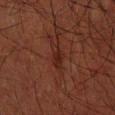Captured during whole-body skin photography for melanoma surveillance; the lesion was not biopsied.
The lesion is located on the right forearm.
A male patient in their 60s.
Captured under cross-polarized illumination.
A 15 mm crop from a total-body photograph taken for skin-cancer surveillance.
Automated image analysis of the tile measured an area of roughly 4.5 mm², an outline eccentricity of about 0.85 (0 = round, 1 = elongated), and two-axis asymmetry of about 0.35. It also reported a lesion color around L≈19 a*≈18 b*≈20 in CIELAB. And it measured a border-irregularity index near 3.5/10, a within-lesion color-variation index near 1.5/10, and peripheral color asymmetry of about 0.5. It also reported a classifier nevus-likeness of about 0/100.